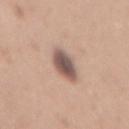image: 15 mm crop, total-body photography; location: the mid back; subject: female, in their 40s; lighting: white-light; lesion diameter: ~4.5 mm (longest diameter).